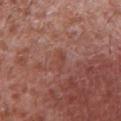Case summary:
* notes: no biopsy performed (imaged during a skin exam)
* anatomic site: the front of the torso
* patient: male, aged 43 to 47
* image source: total-body-photography crop, ~15 mm field of view
* automated metrics: a footprint of about 2.5 mm², a shape eccentricity near 0.9, and a shape-asymmetry score of about 0.4 (0 = symmetric); a nevus-likeness score of about 0/100 and lesion-presence confidence of about 90/100